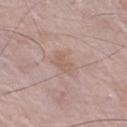No biopsy was performed on this lesion — it was imaged during a full skin examination and was not determined to be concerning.
The tile uses white-light illumination.
A region of skin cropped from a whole-body photographic capture, roughly 15 mm wide.
Located on the left thigh.
An algorithmic analysis of the crop reported a footprint of about 5 mm², a shape eccentricity near 0.9, and a shape-asymmetry score of about 0.35 (0 = symmetric). And it measured a lesion–skin lightness drop of about 6 and a normalized lesion–skin contrast near 5. The analysis additionally found a border-irregularity index near 3.5/10, a color-variation rating of about 2/10, and radial color variation of about 1.
A male patient, in their mid- to late 70s.
The recorded lesion diameter is about 3.5 mm.